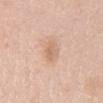The lesion was tiled from a total-body skin photograph and was not biopsied. A female subject roughly 50 years of age. This image is a 15 mm lesion crop taken from a total-body photograph. Located on the mid back.